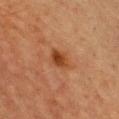Clinical summary: The tile uses cross-polarized illumination. Automated image analysis of the tile measured an average lesion color of about L≈37 a*≈24 b*≈34 (CIELAB), a lesion–skin lightness drop of about 10, and a lesion-to-skin contrast of about 9 (normalized; higher = more distinct). It also reported a classifier nevus-likeness of about 75/100 and a lesion-detection confidence of about 100/100. Measured at roughly 2.5 mm in maximum diameter. The patient is a female aged 63–67. On the chest. Cropped from a total-body skin-imaging series; the visible field is about 15 mm.